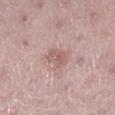Acquisition and patient details:
The lesion's longest dimension is about 3.5 mm. Located on the leg. This image is a 15 mm lesion crop taken from a total-body photograph. The subject is a female aged approximately 45. The total-body-photography lesion software estimated a lesion area of about 5.5 mm², an outline eccentricity of about 0.8 (0 = round, 1 = elongated), and a symmetry-axis asymmetry near 0.2. And it measured a border-irregularity rating of about 2.5/10 and radial color variation of about 1.